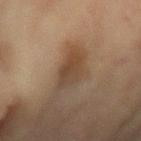No biopsy was performed on this lesion — it was imaged during a full skin examination and was not determined to be concerning.
A female patient roughly 80 years of age.
A lesion tile, about 15 mm wide, cut from a 3D total-body photograph.
The lesion is on the left forearm.
The tile uses cross-polarized illumination.